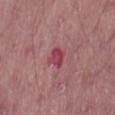Imaged during a routine full-body skin examination; the lesion was not biopsied and no histopathology is available. Imaged with white-light lighting. The subject is a male aged 63 to 67. This image is a 15 mm lesion crop taken from a total-body photograph. The lesion is on the right thigh. Measured at roughly 2.5 mm in maximum diameter.